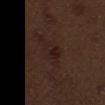Q: Was this lesion biopsied?
A: catalogued during a skin exam; not biopsied
Q: What did automated image analysis measure?
A: an average lesion color of about L≈20 a*≈15 b*≈18 (CIELAB), about 4 CIELAB-L* units darker than the surrounding skin, and a normalized border contrast of about 6
Q: How was the tile lit?
A: white-light
Q: How large is the lesion?
A: ≈4.5 mm
Q: Where on the body is the lesion?
A: the mid back
Q: How was this image acquired?
A: 15 mm crop, total-body photography
Q: Who is the patient?
A: male, aged 68 to 72